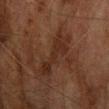Notes:
- follow-up: total-body-photography surveillance lesion; no biopsy
- image: ~15 mm tile from a whole-body skin photo
- anatomic site: the chest
- patient: male, aged 73 to 77
- lesion size: ~7 mm (longest diameter)
- lighting: cross-polarized